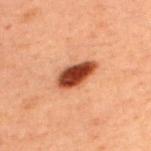Recorded during total-body skin imaging; not selected for excision or biopsy.
The lesion is located on the upper back.
Cropped from a total-body skin-imaging series; the visible field is about 15 mm.
A male patient, in their 60s.